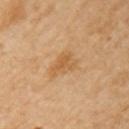| feature | finding |
|---|---|
| workup | imaged on a skin check; not biopsied |
| illumination | cross-polarized illumination |
| TBP lesion metrics | a lesion area of about 6.5 mm² and a symmetry-axis asymmetry near 0.45; about 8 CIELAB-L* units darker than the surrounding skin and a lesion-to-skin contrast of about 6 (normalized; higher = more distinct); a detector confidence of about 100 out of 100 that the crop contains a lesion |
| subject | female, in their 70s |
| image | total-body-photography crop, ~15 mm field of view |
| location | the left upper arm |